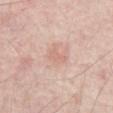Background:
The lesion's longest dimension is about 2.5 mm. The lesion is located on the abdomen. This image is a 15 mm lesion crop taken from a total-body photograph. A patient in their mid-50s. The tile uses cross-polarized illumination.